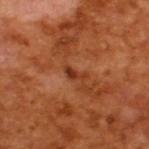No biopsy was performed on this lesion — it was imaged during a full skin examination and was not determined to be concerning. Cropped from a whole-body photographic skin survey; the tile spans about 15 mm. The total-body-photography lesion software estimated an area of roughly 2.5 mm², an eccentricity of roughly 0.9, and a symmetry-axis asymmetry near 0.55. And it measured a mean CIELAB color near L≈36 a*≈29 b*≈35, a lesion–skin lightness drop of about 9, and a lesion-to-skin contrast of about 7.5 (normalized; higher = more distinct). And it measured a color-variation rating of about 0/10. It also reported an automated nevus-likeness rating near 0 out of 100 and a lesion-detection confidence of about 100/100. A male subject aged 63 to 67. The lesion's longest dimension is about 2.5 mm.